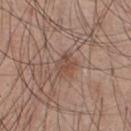On the front of the torso. This is a white-light tile. The recorded lesion diameter is about 3 mm. A male patient approximately 25 years of age. A region of skin cropped from a whole-body photographic capture, roughly 15 mm wide.